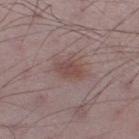biopsy_status: not biopsied; imaged during a skin examination